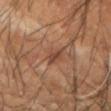Clinical impression:
No biopsy was performed on this lesion — it was imaged during a full skin examination and was not determined to be concerning.
Acquisition and patient details:
The recorded lesion diameter is about 4.5 mm. Located on the left forearm. A male subject in their mid-50s. A roughly 15 mm field-of-view crop from a total-body skin photograph. An algorithmic analysis of the crop reported a footprint of about 4.5 mm² and two-axis asymmetry of about 0.25.